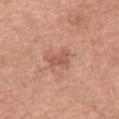Q: Is there a histopathology result?
A: imaged on a skin check; not biopsied
Q: Where on the body is the lesion?
A: the upper back
Q: How was the tile lit?
A: white-light
Q: Patient demographics?
A: female, aged around 60
Q: What is the imaging modality?
A: total-body-photography crop, ~15 mm field of view
Q: How large is the lesion?
A: about 3 mm